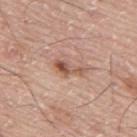Clinical impression: The lesion was photographed on a routine skin check and not biopsied; there is no pathology result. Image and clinical context: Approximately 4 mm at its widest. A 15 mm crop from a total-body photograph taken for skin-cancer surveillance. The tile uses white-light illumination. The lesion is located on the back. A male patient, in their mid-70s.